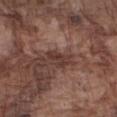• image: ~15 mm tile from a whole-body skin photo
• subject: male, aged 73 to 77
• location: the arm